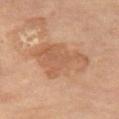Impression: No biopsy was performed on this lesion — it was imaged during a full skin examination and was not determined to be concerning. Acquisition and patient details: Longest diameter approximately 7 mm. Imaged with cross-polarized lighting. A female subject, aged approximately 70. The lesion is on the right thigh. A close-up tile cropped from a whole-body skin photograph, about 15 mm across.